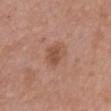Case summary:
– notes — imaged on a skin check; not biopsied